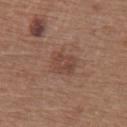Findings:
• notes · catalogued during a skin exam; not biopsied
• subject · female, aged 63–67
• anatomic site · the right upper arm
• acquisition · ~15 mm crop, total-body skin-cancer survey
• automated lesion analysis · roughly 7 lightness units darker than nearby skin and a normalized lesion–skin contrast near 6
• lesion size · ~3.5 mm (longest diameter)